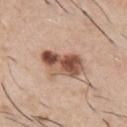Q: Was this lesion biopsied?
A: no biopsy performed (imaged during a skin exam)
Q: Illumination type?
A: white-light illumination
Q: Lesion size?
A: ≈5 mm
Q: Automated lesion metrics?
A: an area of roughly 11 mm², an eccentricity of roughly 0.85, and two-axis asymmetry of about 0.25; a classifier nevus-likeness of about 95/100
Q: Where on the body is the lesion?
A: the chest
Q: How was this image acquired?
A: total-body-photography crop, ~15 mm field of view
Q: Patient demographics?
A: male, aged around 60